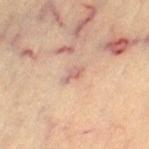<lesion>
<biopsy_status>not biopsied; imaged during a skin examination</biopsy_status>
<patient>
  <sex>female</sex>
  <age_approx>65</age_approx>
</patient>
<site>leg</site>
<automated_metrics>
  <eccentricity>0.9</eccentricity>
  <shape_asymmetry>0.35</shape_asymmetry>
  <lesion_detection_confidence_0_100>85</lesion_detection_confidence_0_100>
</automated_metrics>
<image>
  <source>total-body photography crop</source>
  <field_of_view_mm>15</field_of_view_mm>
</image>
</lesion>